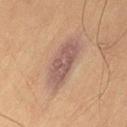Imaged during a routine full-body skin examination; the lesion was not biopsied and no histopathology is available. The total-body-photography lesion software estimated a mean CIELAB color near L≈44 a*≈16 b*≈20 and a lesion-to-skin contrast of about 9 (normalized; higher = more distinct). And it measured a border-irregularity index near 2.5/10, internal color variation of about 2.5 on a 0–10 scale, and a peripheral color-asymmetry measure near 1. On the left thigh. A male subject, aged around 70. A lesion tile, about 15 mm wide, cut from a 3D total-body photograph. This is a cross-polarized tile.